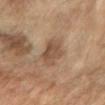The lesion was photographed on a routine skin check and not biopsied; there is no pathology result.
Captured under cross-polarized illumination.
The patient is a female about 70 years old.
This image is a 15 mm lesion crop taken from a total-body photograph.
The lesion's longest dimension is about 5 mm.
From the right forearm.